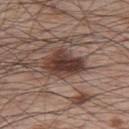Q: Is there a histopathology result?
A: no biopsy performed (imaged during a skin exam)
Q: How was the tile lit?
A: white-light illumination
Q: Lesion size?
A: ≈5 mm
Q: What is the imaging modality?
A: ~15 mm crop, total-body skin-cancer survey
Q: What are the patient's age and sex?
A: male, roughly 65 years of age
Q: Where on the body is the lesion?
A: the upper back